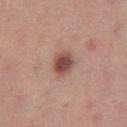Clinical impression: The lesion was photographed on a routine skin check and not biopsied; there is no pathology result. Context: About 3 mm across. A roughly 15 mm field-of-view crop from a total-body skin photograph. Captured under white-light illumination. The lesion is on the left thigh. A female patient, aged approximately 25.